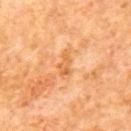  biopsy_status: not biopsied; imaged during a skin examination
  automated_metrics:
    area_mm2_approx: 3.5
    eccentricity: 0.85
    shape_asymmetry: 0.4
    cielab_L: 62
    cielab_a: 26
    cielab_b: 45
    vs_skin_contrast_norm: 6.0
    border_irregularity_0_10: 4.0
    color_variation_0_10: 2.0
    nevus_likeness_0_100: 0
    lesion_detection_confidence_0_100: 100
  lighting: cross-polarized
  image:
    source: total-body photography crop
    field_of_view_mm: 15
  lesion_size:
    long_diameter_mm_approx: 2.5
  site: mid back
  patient:
    sex: male
    age_approx: 65